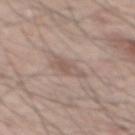{"biopsy_status": "not biopsied; imaged during a skin examination", "image": {"source": "total-body photography crop", "field_of_view_mm": 15}, "patient": {"sex": "male", "age_approx": 55}, "site": "mid back", "lesion_size": {"long_diameter_mm_approx": 3.5}, "lighting": "white-light"}